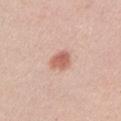{
  "biopsy_status": "not biopsied; imaged during a skin examination",
  "patient": {
    "sex": "female",
    "age_approx": 55
  },
  "lesion_size": {
    "long_diameter_mm_approx": 3.0
  },
  "image": {
    "source": "total-body photography crop",
    "field_of_view_mm": 15
  },
  "lighting": "white-light",
  "automated_metrics": {
    "vs_skin_contrast_norm": 7.5,
    "nevus_likeness_0_100": 95
  },
  "site": "left upper arm"
}